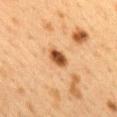Recorded during total-body skin imaging; not selected for excision or biopsy. A female subject, roughly 40 years of age. The recorded lesion diameter is about 3 mm. Cropped from a whole-body photographic skin survey; the tile spans about 15 mm. Located on the mid back. The tile uses cross-polarized illumination. Automated image analysis of the tile measured an average lesion color of about L≈44 a*≈21 b*≈35 (CIELAB) and a lesion–skin lightness drop of about 16. The software also gave a border-irregularity rating of about 1.5/10, a within-lesion color-variation index near 4/10, and radial color variation of about 1.5. And it measured lesion-presence confidence of about 100/100.